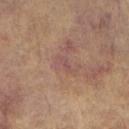- tile lighting — white-light
- automated metrics — a footprint of about 4 mm², a shape eccentricity near 0.85, and two-axis asymmetry of about 0.4; a border-irregularity rating of about 5/10, a within-lesion color-variation index near 0.5/10, and a peripheral color-asymmetry measure near 0
- patient — male, aged around 60
- acquisition — 15 mm crop, total-body photography
- location — the right forearm